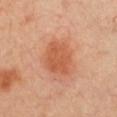Case summary:
- biopsy status — no biopsy performed (imaged during a skin exam)
- image — ~15 mm crop, total-body skin-cancer survey
- body site — the chest
- patient — female, aged 38–42
- lighting — cross-polarized illumination
- diameter — ≈4.5 mm
- TBP lesion metrics — a nevus-likeness score of about 95/100 and a detector confidence of about 100 out of 100 that the crop contains a lesion